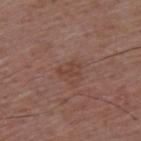notes — catalogued during a skin exam; not biopsied
patient — male, aged 53 to 57
body site — the upper back
diameter — ~2.5 mm (longest diameter)
image — total-body-photography crop, ~15 mm field of view
image-analysis metrics — a footprint of about 2.5 mm², a shape eccentricity near 0.8, and a shape-asymmetry score of about 0.55 (0 = symmetric); a border-irregularity index near 8.5/10, a color-variation rating of about 0/10, and radial color variation of about 0